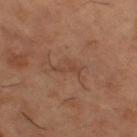• biopsy status · no biopsy performed (imaged during a skin exam)
• TBP lesion metrics · a lesion–skin lightness drop of about 6 and a normalized border contrast of about 4.5; a detector confidence of about 65 out of 100 that the crop contains a lesion
• diameter · ~3.5 mm (longest diameter)
• site · the right thigh
• image · total-body-photography crop, ~15 mm field of view
• patient · male, aged 63–67